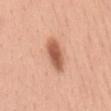{"biopsy_status": "not biopsied; imaged during a skin examination", "patient": {"sex": "female", "age_approx": 35}, "image": {"source": "total-body photography crop", "field_of_view_mm": 15}, "lesion_size": {"long_diameter_mm_approx": 4.5}, "lighting": "white-light", "site": "mid back", "automated_metrics": {"eccentricity": 0.9, "shape_asymmetry": 0.2, "cielab_L": 58, "cielab_a": 26, "cielab_b": 33, "vs_skin_darker_L": 15.0, "vs_skin_contrast_norm": 9.5, "nevus_likeness_0_100": 100, "lesion_detection_confidence_0_100": 100}}